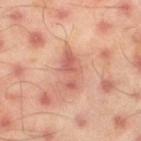{
  "biopsy_status": "not biopsied; imaged during a skin examination",
  "image": {
    "source": "total-body photography crop",
    "field_of_view_mm": 15
  },
  "site": "left thigh",
  "lighting": "cross-polarized",
  "automated_metrics": {
    "area_mm2_approx": 7.5,
    "eccentricity": 0.9
  },
  "lesion_size": {
    "long_diameter_mm_approx": 4.5
  },
  "patient": {
    "sex": "male",
    "age_approx": 45
  }
}